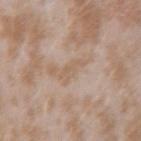{"biopsy_status": "not biopsied; imaged during a skin examination"}